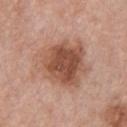follow-up: imaged on a skin check; not biopsied | illumination: white-light illumination | patient: male, aged approximately 60 | site: the front of the torso | lesion diameter: ~6.5 mm (longest diameter) | acquisition: total-body-photography crop, ~15 mm field of view.